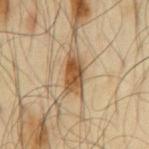Recorded during total-body skin imaging; not selected for excision or biopsy.
The tile uses cross-polarized illumination.
A male subject, approximately 65 years of age.
On the back.
A close-up tile cropped from a whole-body skin photograph, about 15 mm across.
The lesion's longest dimension is about 4.5 mm.